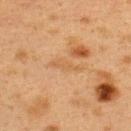<record>
  <patient>
    <sex>female</sex>
    <age_approx>40</age_approx>
  </patient>
  <image>
    <source>total-body photography crop</source>
    <field_of_view_mm>15</field_of_view_mm>
  </image>
  <lesion_size>
    <long_diameter_mm_approx>4.0</long_diameter_mm_approx>
  </lesion_size>
  <automated_metrics>
    <area_mm2_approx>4.5</area_mm2_approx>
    <eccentricity>0.95</eccentricity>
    <shape_asymmetry>0.35</shape_asymmetry>
    <cielab_L>49</cielab_L>
    <cielab_a>17</cielab_a>
    <cielab_b>34</cielab_b>
    <vs_skin_darker_L>5.0</vs_skin_darker_L>
    <vs_skin_contrast_norm>4.5</vs_skin_contrast_norm>
    <nevus_likeness_0_100>0</nevus_likeness_0_100>
    <lesion_detection_confidence_0_100>95</lesion_detection_confidence_0_100>
  </automated_metrics>
  <lighting>cross-polarized</lighting>
  <site>back</site>
</record>